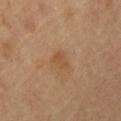Part of a total-body skin-imaging series; this lesion was reviewed on a skin check and was not flagged for biopsy.
A male patient in their mid- to late 60s.
A close-up tile cropped from a whole-body skin photograph, about 15 mm across.
The lesion is on the chest.